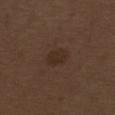Impression: Part of a total-body skin-imaging series; this lesion was reviewed on a skin check and was not flagged for biopsy. Image and clinical context: This is a white-light tile. The lesion is located on the right thigh. The recorded lesion diameter is about 3 mm. A male patient, aged 68 to 72. A region of skin cropped from a whole-body photographic capture, roughly 15 mm wide.